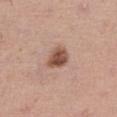Impression: Part of a total-body skin-imaging series; this lesion was reviewed on a skin check and was not flagged for biopsy. Image and clinical context: A male subject aged 63 to 67. Located on the right thigh. Automated image analysis of the tile measured a lesion area of about 6.5 mm², an outline eccentricity of about 0.35 (0 = round, 1 = elongated), and a shape-asymmetry score of about 0.2 (0 = symmetric). The software also gave a border-irregularity rating of about 2/10. And it measured an automated nevus-likeness rating near 95 out of 100 and a lesion-detection confidence of about 100/100. This image is a 15 mm lesion crop taken from a total-body photograph. Imaged with white-light lighting.